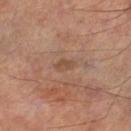notes = catalogued during a skin exam; not biopsied | anatomic site = the left lower leg | illumination = cross-polarized | patient = male, roughly 55 years of age | image source = ~15 mm tile from a whole-body skin photo | lesion size = ≈2.5 mm.